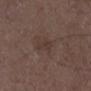{
  "biopsy_status": "not biopsied; imaged during a skin examination",
  "lesion_size": {
    "long_diameter_mm_approx": 2.5
  },
  "site": "right lower leg",
  "automated_metrics": {
    "shape_asymmetry": 0.45,
    "border_irregularity_0_10": 4.5,
    "color_variation_0_10": 1.0,
    "peripheral_color_asymmetry": 0.5
  },
  "patient": {
    "sex": "male",
    "age_approx": 75
  },
  "image": {
    "source": "total-body photography crop",
    "field_of_view_mm": 15
  },
  "lighting": "white-light"
}